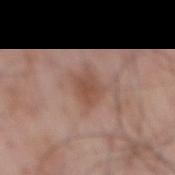Assessment:
Captured during whole-body skin photography for melanoma surveillance; the lesion was not biopsied.
Context:
A roughly 15 mm field-of-view crop from a total-body skin photograph. On the mid back. The patient is a male in their 70s. This is a white-light tile.